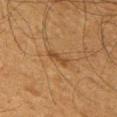Assessment:
No biopsy was performed on this lesion — it was imaged during a full skin examination and was not determined to be concerning.
Acquisition and patient details:
From the arm. A male subject roughly 60 years of age. A region of skin cropped from a whole-body photographic capture, roughly 15 mm wide. Automated image analysis of the tile measured an average lesion color of about L≈43 a*≈19 b*≈35 (CIELAB), roughly 8 lightness units darker than nearby skin, and a normalized lesion–skin contrast near 6.5.